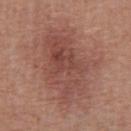Case summary:
• follow-up · imaged on a skin check; not biopsied
• illumination · white-light illumination
• imaging modality · ~15 mm crop, total-body skin-cancer survey
• site · the abdomen
• subject · male, aged 63 to 67
• lesion diameter · ≈8.5 mm